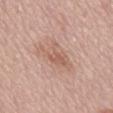notes=catalogued during a skin exam; not biopsied
image source=~15 mm tile from a whole-body skin photo
patient=female, approximately 65 years of age
body site=the mid back